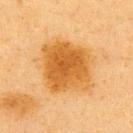<record>
  <biopsy_status>not biopsied; imaged during a skin examination</biopsy_status>
</record>